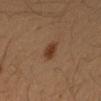<lesion>
  <patient>
    <sex>female</sex>
    <age_approx>40</age_approx>
  </patient>
  <lighting>cross-polarized</lighting>
  <lesion_size>
    <long_diameter_mm_approx>2.5</long_diameter_mm_approx>
  </lesion_size>
  <automated_metrics>
    <nevus_likeness_0_100>95</nevus_likeness_0_100>
    <lesion_detection_confidence_0_100>100</lesion_detection_confidence_0_100>
  </automated_metrics>
  <image>
    <source>total-body photography crop</source>
    <field_of_view_mm>15</field_of_view_mm>
  </image>
  <site>right forearm</site>
</lesion>